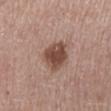Impression: Part of a total-body skin-imaging series; this lesion was reviewed on a skin check and was not flagged for biopsy. Image and clinical context: The patient is a male in their mid-50s. Cropped from a whole-body photographic skin survey; the tile spans about 15 mm. Approximately 3.5 mm at its widest. The total-body-photography lesion software estimated a border-irregularity rating of about 2/10 and peripheral color asymmetry of about 1. Captured under white-light illumination.